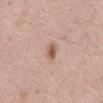{"biopsy_status": "not biopsied; imaged during a skin examination", "image": {"source": "total-body photography crop", "field_of_view_mm": 15}, "site": "abdomen", "automated_metrics": {"area_mm2_approx": 3.0, "eccentricity": 0.8, "shape_asymmetry": 0.25}, "lighting": "white-light", "patient": {"sex": "male", "age_approx": 55}}